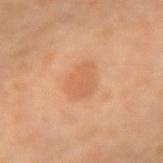{"biopsy_status": "not biopsied; imaged during a skin examination"}